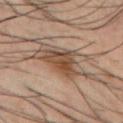  biopsy_status: not biopsied; imaged during a skin examination
  image:
    source: total-body photography crop
    field_of_view_mm: 15
  patient:
    sex: male
    age_approx: 40
  lesion_size:
    long_diameter_mm_approx: 8.0
  site: chest
  lighting: cross-polarized